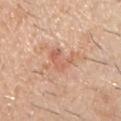Context:
The recorded lesion diameter is about 3.5 mm. From the front of the torso. The subject is a male approximately 60 years of age. Captured under white-light illumination. The total-body-photography lesion software estimated an area of roughly 5.5 mm² and a shape eccentricity near 0.8. It also reported roughly 8 lightness units darker than nearby skin and a lesion-to-skin contrast of about 5.5 (normalized; higher = more distinct). The analysis additionally found border irregularity of about 5 on a 0–10 scale, a color-variation rating of about 3/10, and peripheral color asymmetry of about 0.5. And it measured a classifier nevus-likeness of about 0/100 and lesion-presence confidence of about 100/100. A 15 mm close-up extracted from a 3D total-body photography capture.